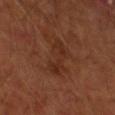Impression:
The lesion was tiled from a total-body skin photograph and was not biopsied.